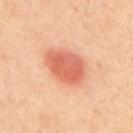{"biopsy_status": "not biopsied; imaged during a skin examination", "site": "upper back", "patient": {"sex": "male", "age_approx": 40}, "image": {"source": "total-body photography crop", "field_of_view_mm": 15}, "lighting": "cross-polarized"}